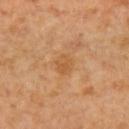– workup · total-body-photography surveillance lesion; no biopsy
– size · ~2.5 mm (longest diameter)
– imaging modality · ~15 mm crop, total-body skin-cancer survey
– automated lesion analysis · an average lesion color of about L≈53 a*≈22 b*≈39 (CIELAB), roughly 6 lightness units darker than nearby skin, and a normalized border contrast of about 5.5; a border-irregularity rating of about 2/10, a color-variation rating of about 2.5/10, and a peripheral color-asymmetry measure near 1; a classifier nevus-likeness of about 0/100 and lesion-presence confidence of about 100/100
– subject · female, aged around 50
– location · the arm